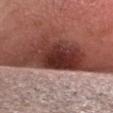Impression: Part of a total-body skin-imaging series; this lesion was reviewed on a skin check and was not flagged for biopsy.